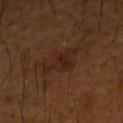notes: total-body-photography surveillance lesion; no biopsy
tile lighting: cross-polarized
subject: male, aged around 65
size: about 4 mm
site: the right forearm
imaging modality: 15 mm crop, total-body photography